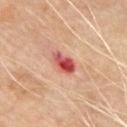lighting=cross-polarized
patient=male, approximately 65 years of age
anatomic site=the front of the torso
imaging modality=~15 mm tile from a whole-body skin photo
automated metrics=a lesion color around L≈51 a*≈35 b*≈28 in CIELAB and roughly 15 lightness units darker than nearby skin
lesion size=about 3.5 mm